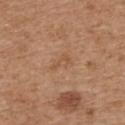Clinical impression: The lesion was photographed on a routine skin check and not biopsied; there is no pathology result. Clinical summary: A lesion tile, about 15 mm wide, cut from a 3D total-body photograph. The lesion is on the upper back. The lesion's longest dimension is about 2.5 mm. The subject is a male aged around 70. Automated tile analysis of the lesion measured a footprint of about 2.5 mm², a shape eccentricity near 0.9, and two-axis asymmetry of about 0.45. The software also gave an average lesion color of about L≈53 a*≈21 b*≈34 (CIELAB), about 6 CIELAB-L* units darker than the surrounding skin, and a lesion-to-skin contrast of about 4.5 (normalized; higher = more distinct). It also reported border irregularity of about 4.5 on a 0–10 scale and a peripheral color-asymmetry measure near 0. And it measured a classifier nevus-likeness of about 0/100 and a detector confidence of about 100 out of 100 that the crop contains a lesion.